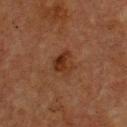Impression: Recorded during total-body skin imaging; not selected for excision or biopsy. Acquisition and patient details: A lesion tile, about 15 mm wide, cut from a 3D total-body photograph. A male subject, in their mid- to late 70s. The lesion is located on the chest.